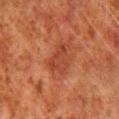<record>
<biopsy_status>not biopsied; imaged during a skin examination</biopsy_status>
<image>
  <source>total-body photography crop</source>
  <field_of_view_mm>15</field_of_view_mm>
</image>
<site>right lower leg</site>
<patient>
  <sex>male</sex>
  <age_approx>80</age_approx>
</patient>
</record>